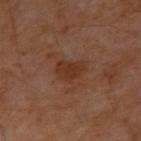biopsy status: no biopsy performed (imaged during a skin exam)
illumination: cross-polarized illumination
image source: ~15 mm tile from a whole-body skin photo
size: ~3.5 mm (longest diameter)
subject: male, roughly 55 years of age
automated lesion analysis: a lesion area of about 6 mm², a shape eccentricity near 0.7, and a shape-asymmetry score of about 0.3 (0 = symmetric); roughly 7 lightness units darker than nearby skin and a lesion-to-skin contrast of about 7.5 (normalized; higher = more distinct)
anatomic site: the right upper arm